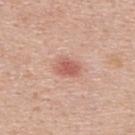biopsy_status: not biopsied; imaged during a skin examination
lesion_size:
  long_diameter_mm_approx: 3.0
automated_metrics:
  cielab_L: 59
  cielab_a: 26
  cielab_b: 28
  vs_skin_darker_L: 11.0
  vs_skin_contrast_norm: 7.0
  nevus_likeness_0_100: 85
  lesion_detection_confidence_0_100: 100
image:
  source: total-body photography crop
  field_of_view_mm: 15
patient:
  sex: male
  age_approx: 40
site: upper back